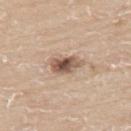Notes:
– biopsy status · catalogued during a skin exam; not biopsied
– site · the back
– lesion size · ~3.5 mm (longest diameter)
– image · ~15 mm tile from a whole-body skin photo
– patient · male, aged 58 to 62